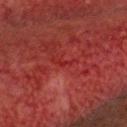No biopsy was performed on this lesion — it was imaged during a full skin examination and was not determined to be concerning. Located on the head or neck. A 15 mm close-up tile from a total-body photography series done for melanoma screening. About 1.5 mm across. A male patient, in their 50s.